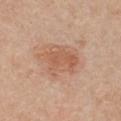Recorded during total-body skin imaging; not selected for excision or biopsy.
The tile uses white-light illumination.
The patient is a female about 45 years old.
On the front of the torso.
This image is a 15 mm lesion crop taken from a total-body photograph.
Measured at roughly 5 mm in maximum diameter.